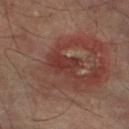Captured during whole-body skin photography for melanoma surveillance; the lesion was not biopsied.
Imaged with cross-polarized lighting.
Approximately 3.5 mm at its widest.
This image is a 15 mm lesion crop taken from a total-body photograph.
The lesion-visualizer software estimated a footprint of about 7 mm², an outline eccentricity of about 0.75 (0 = round, 1 = elongated), and a shape-asymmetry score of about 0.3 (0 = symmetric). The software also gave a classifier nevus-likeness of about 0/100 and lesion-presence confidence of about 90/100.
On the left lower leg.
A male patient, in their mid- to late 70s.